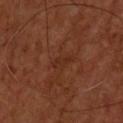The lesion is on the upper back.
A male subject about 55 years old.
A 15 mm crop from a total-body photograph taken for skin-cancer surveillance.
Automated tile analysis of the lesion measured an area of roughly 2.5 mm², an outline eccentricity of about 0.9 (0 = round, 1 = elongated), and two-axis asymmetry of about 0.35. It also reported a lesion color around L≈25 a*≈21 b*≈26 in CIELAB, a lesion–skin lightness drop of about 4, and a normalized border contrast of about 5. The software also gave a border-irregularity index near 4/10, a within-lesion color-variation index near 0/10, and radial color variation of about 0. The software also gave a lesion-detection confidence of about 100/100.